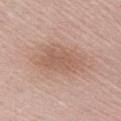Assessment: Recorded during total-body skin imaging; not selected for excision or biopsy. Background: The tile uses white-light illumination. The recorded lesion diameter is about 6 mm. A region of skin cropped from a whole-body photographic capture, roughly 15 mm wide. The total-body-photography lesion software estimated a lesion area of about 18 mm², an eccentricity of roughly 0.75, and a shape-asymmetry score of about 0.25 (0 = symmetric). The software also gave an automated nevus-likeness rating near 0 out of 100 and a detector confidence of about 100 out of 100 that the crop contains a lesion. A male subject roughly 65 years of age. The lesion is on the right upper arm.